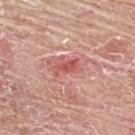Impression: Captured during whole-body skin photography for melanoma surveillance; the lesion was not biopsied. Image and clinical context: The lesion is located on the upper back. A male subject, aged 63–67. The tile uses white-light illumination. A close-up tile cropped from a whole-body skin photograph, about 15 mm across. Automated image analysis of the tile measured an average lesion color of about L≈55 a*≈33 b*≈25 (CIELAB), roughly 10 lightness units darker than nearby skin, and a normalized border contrast of about 7. The software also gave an automated nevus-likeness rating near 0 out of 100 and lesion-presence confidence of about 100/100.